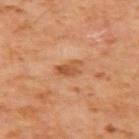The lesion was tiled from a total-body skin photograph and was not biopsied. The lesion's longest dimension is about 3 mm. Imaged with cross-polarized lighting. The lesion-visualizer software estimated a mean CIELAB color near L≈50 a*≈24 b*≈37, roughly 9 lightness units darker than nearby skin, and a normalized lesion–skin contrast near 7.5. A male subject, aged around 60. The lesion is on the left upper arm. A close-up tile cropped from a whole-body skin photograph, about 15 mm across.